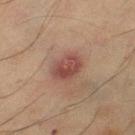The lesion is on the left lower leg.
The patient is a female about 55 years old.
A lesion tile, about 15 mm wide, cut from a 3D total-body photograph.